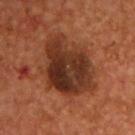No biopsy was performed on this lesion — it was imaged during a full skin examination and was not determined to be concerning.